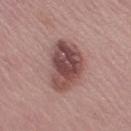notes — catalogued during a skin exam; not biopsied | illumination — white-light | body site — the left thigh | imaging modality — ~15 mm tile from a whole-body skin photo | size — ~7 mm (longest diameter) | image-analysis metrics — an area of roughly 19 mm², an eccentricity of roughly 0.85, and two-axis asymmetry of about 0.25; a lesion-to-skin contrast of about 10 (normalized; higher = more distinct); internal color variation of about 7 on a 0–10 scale; a classifier nevus-likeness of about 65/100 and a detector confidence of about 100 out of 100 that the crop contains a lesion | patient — female, aged around 65.